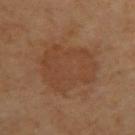Part of a total-body skin-imaging series; this lesion was reviewed on a skin check and was not flagged for biopsy. The total-body-photography lesion software estimated a lesion area of about 28 mm² and an outline eccentricity of about 0.55 (0 = round, 1 = elongated). The analysis additionally found a normalized lesion–skin contrast near 5. The analysis additionally found a border-irregularity rating of about 3/10, a within-lesion color-variation index near 2.5/10, and radial color variation of about 0.5. The analysis additionally found a classifier nevus-likeness of about 85/100 and lesion-presence confidence of about 100/100. The lesion is on the upper back. This is a cross-polarized tile. A 15 mm close-up extracted from a 3D total-body photography capture. A female patient about 70 years old. Measured at roughly 6.5 mm in maximum diameter.